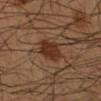{
  "patient": {
    "sex": "male",
    "age_approx": 55
  },
  "lesion_size": {
    "long_diameter_mm_approx": 3.5
  },
  "site": "arm",
  "image": {
    "source": "total-body photography crop",
    "field_of_view_mm": 15
  },
  "automated_metrics": {
    "area_mm2_approx": 7.5,
    "eccentricity": 0.7,
    "shape_asymmetry": 0.2,
    "nevus_likeness_0_100": 90
  },
  "lighting": "cross-polarized"
}